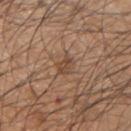Notes:
* notes: imaged on a skin check; not biopsied
* lighting: white-light
* anatomic site: the right upper arm
* patient: male, roughly 45 years of age
* image: 15 mm crop, total-body photography